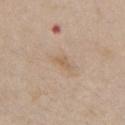Captured during whole-body skin photography for melanoma surveillance; the lesion was not biopsied. Measured at roughly 2.5 mm in maximum diameter. A close-up tile cropped from a whole-body skin photograph, about 15 mm across. The subject is a female aged around 70. The lesion is located on the chest.